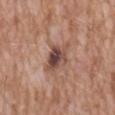biopsy_status: not biopsied; imaged during a skin examination
image:
  source: total-body photography crop
  field_of_view_mm: 15
site: abdomen
lesion_size:
  long_diameter_mm_approx: 4.5
patient:
  sex: male
  age_approx: 60
lighting: white-light